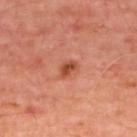Q: Was a biopsy performed?
A: no biopsy performed (imaged during a skin exam)